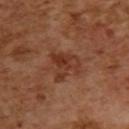biopsy status — imaged on a skin check; not biopsied
location — the upper back
lighting — cross-polarized
subject — female, aged approximately 55
automated lesion analysis — a lesion color around L≈37 a*≈24 b*≈31 in CIELAB, about 8 CIELAB-L* units darker than the surrounding skin, and a lesion-to-skin contrast of about 7 (normalized; higher = more distinct); a classifier nevus-likeness of about 5/100 and a detector confidence of about 100 out of 100 that the crop contains a lesion
image — total-body-photography crop, ~15 mm field of view
lesion diameter — ≈4 mm